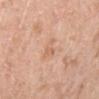notes = total-body-photography surveillance lesion; no biopsy | automated lesion analysis = an area of roughly 3.5 mm² and a shape eccentricity near 0.7; a border-irregularity index near 3.5/10, a color-variation rating of about 1.5/10, and peripheral color asymmetry of about 0.5; a classifier nevus-likeness of about 0/100 and lesion-presence confidence of about 100/100 | acquisition = total-body-photography crop, ~15 mm field of view | subject = female, aged 68–72 | anatomic site = the right lower leg | size = about 3 mm.